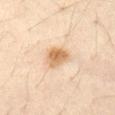workup: imaged on a skin check; not biopsied
acquisition: ~15 mm crop, total-body skin-cancer survey
illumination: cross-polarized illumination
lesion size: about 3.5 mm
anatomic site: the abdomen
patient: female, roughly 35 years of age